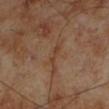<case>
  <patient>
    <sex>male</sex>
    <age_approx>70</age_approx>
  </patient>
  <image>
    <source>total-body photography crop</source>
    <field_of_view_mm>15</field_of_view_mm>
  </image>
  <site>left lower leg</site>
  <automated_metrics>
    <area_mm2_approx>2.5</area_mm2_approx>
    <eccentricity>0.95</eccentricity>
    <shape_asymmetry>0.35</shape_asymmetry>
    <vs_skin_darker_L>6.0</vs_skin_darker_L>
    <nevus_likeness_0_100>0</nevus_likeness_0_100>
  </automated_metrics>
</case>